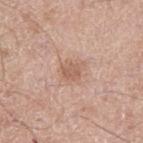Notes:
• workup — catalogued during a skin exam; not biopsied
• image-analysis metrics — a within-lesion color-variation index near 2/10 and peripheral color asymmetry of about 0.5; a classifier nevus-likeness of about 25/100 and a detector confidence of about 100 out of 100 that the crop contains a lesion
• patient — male, about 65 years old
• lesion diameter — ~3 mm (longest diameter)
• anatomic site — the leg
• lighting — white-light
• imaging modality — total-body-photography crop, ~15 mm field of view